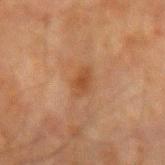Imaged during a routine full-body skin examination; the lesion was not biopsied and no histopathology is available. The lesion-visualizer software estimated a nevus-likeness score of about 35/100. The lesion is on the left forearm. A male subject in their mid- to late 60s. Cropped from a total-body skin-imaging series; the visible field is about 15 mm. The recorded lesion diameter is about 3 mm.